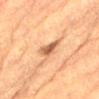acquisition: total-body-photography crop, ~15 mm field of view
location: the lower back
illumination: cross-polarized illumination
subject: male, aged around 85 or older